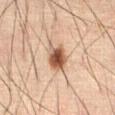  biopsy_status: not biopsied; imaged during a skin examination
  patient:
    sex: male
    age_approx: 60
  lighting: cross-polarized
  image:
    source: total-body photography crop
    field_of_view_mm: 15
  lesion_size:
    long_diameter_mm_approx: 3.5
  automated_metrics:
    nevus_likeness_0_100: 100